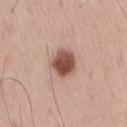{
  "biopsy_status": "not biopsied; imaged during a skin examination",
  "image": {
    "source": "total-body photography crop",
    "field_of_view_mm": 15
  },
  "patient": {
    "sex": "male",
    "age_approx": 55
  },
  "lighting": "white-light",
  "site": "right thigh"
}